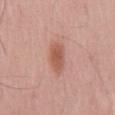tile lighting = white-light illumination | size = ≈4 mm | patient = male, in their 40s | body site = the mid back | image source = 15 mm crop, total-body photography | automated lesion analysis = a footprint of about 7 mm², a shape eccentricity near 0.85, and two-axis asymmetry of about 0.2; a mean CIELAB color near L≈56 a*≈25 b*≈29, about 10 CIELAB-L* units darker than the surrounding skin, and a normalized lesion–skin contrast near 7.5; a nevus-likeness score of about 100/100 and lesion-presence confidence of about 100/100.